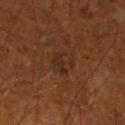<case>
<biopsy_status>not biopsied; imaged during a skin examination</biopsy_status>
<image>
  <source>total-body photography crop</source>
  <field_of_view_mm>15</field_of_view_mm>
</image>
<site>left lower leg</site>
<patient>
  <sex>male</sex>
  <age_approx>60</age_approx>
</patient>
<lighting>cross-polarized</lighting>
<lesion_size>
  <long_diameter_mm_approx>3.5</long_diameter_mm_approx>
</lesion_size>
</case>